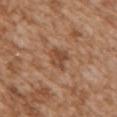Captured during whole-body skin photography for melanoma surveillance; the lesion was not biopsied. Longest diameter approximately 3 mm. The lesion is located on the chest. The tile uses white-light illumination. A roughly 15 mm field-of-view crop from a total-body skin photograph. The patient is a female approximately 30 years of age.